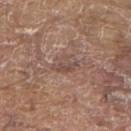workup=catalogued during a skin exam; not biopsied | location=the upper back | lesion size=about 3.5 mm | subject=male, aged 78 to 82 | imaging modality=~15 mm crop, total-body skin-cancer survey | image-analysis metrics=a footprint of about 4.5 mm², an eccentricity of roughly 0.8, and a symmetry-axis asymmetry near 0.35; a lesion color around L≈48 a*≈18 b*≈23 in CIELAB, about 8 CIELAB-L* units darker than the surrounding skin, and a normalized lesion–skin contrast near 6.